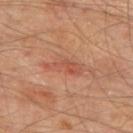A male patient, aged approximately 65. The lesion is on the mid back. This is a cross-polarized tile. Cropped from a total-body skin-imaging series; the visible field is about 15 mm. An algorithmic analysis of the crop reported a shape eccentricity near 0.9 and a symmetry-axis asymmetry near 0.5. The analysis additionally found a lesion color around L≈51 a*≈27 b*≈32 in CIELAB, about 7 CIELAB-L* units darker than the surrounding skin, and a normalized lesion–skin contrast near 5.5. It also reported border irregularity of about 6.5 on a 0–10 scale, a within-lesion color-variation index near 2/10, and a peripheral color-asymmetry measure near 0.5. The analysis additionally found a classifier nevus-likeness of about 0/100 and a detector confidence of about 100 out of 100 that the crop contains a lesion.